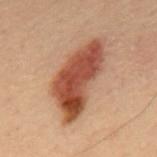Findings:
- patient — male, in their mid- to late 50s
- size — about 9 mm
- TBP lesion metrics — a footprint of about 25 mm², a shape eccentricity near 0.9, and two-axis asymmetry of about 0.35; an automated nevus-likeness rating near 100 out of 100 and a detector confidence of about 100 out of 100 that the crop contains a lesion
- acquisition — 15 mm crop, total-body photography
- location — the mid back
- lighting — cross-polarized